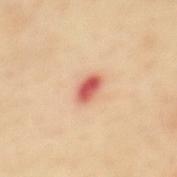Captured during whole-body skin photography for melanoma surveillance; the lesion was not biopsied. Located on the mid back. This image is a 15 mm lesion crop taken from a total-body photograph. Automated image analysis of the tile measured an eccentricity of roughly 0.8 and two-axis asymmetry of about 0.2. The analysis additionally found a mean CIELAB color near L≈62 a*≈33 b*≈33 and a normalized border contrast of about 10. The analysis additionally found a nevus-likeness score of about 0/100 and a detector confidence of about 100 out of 100 that the crop contains a lesion. The tile uses cross-polarized illumination. The patient is a female approximately 40 years of age.